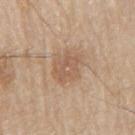No biopsy was performed on this lesion — it was imaged during a full skin examination and was not determined to be concerning. A lesion tile, about 15 mm wide, cut from a 3D total-body photograph. A male subject, roughly 80 years of age. The lesion is on the right upper arm.